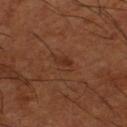Clinical impression:
This lesion was catalogued during total-body skin photography and was not selected for biopsy.
Clinical summary:
The lesion-visualizer software estimated a lesion area of about 4 mm², a shape eccentricity near 0.75, and a shape-asymmetry score of about 0.2 (0 = symmetric). And it measured a lesion color around L≈32 a*≈22 b*≈29 in CIELAB, roughly 5 lightness units darker than nearby skin, and a normalized border contrast of about 5. The software also gave a border-irregularity rating of about 2/10, a color-variation rating of about 3/10, and peripheral color asymmetry of about 1. Cropped from a whole-body photographic skin survey; the tile spans about 15 mm. From the right lower leg. Captured under cross-polarized illumination. A male patient roughly 65 years of age. About 3 mm across.